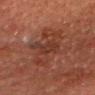– notes — total-body-photography surveillance lesion; no biopsy
– lighting — cross-polarized
– size — ~3.5 mm (longest diameter)
– image — 15 mm crop, total-body photography
– site — the head or neck
– patient — male, roughly 80 years of age